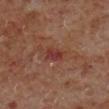Q: Is there a histopathology result?
A: imaged on a skin check; not biopsied
Q: What are the patient's age and sex?
A: male, about 60 years old
Q: Automated lesion metrics?
A: a classifier nevus-likeness of about 0/100 and a lesion-detection confidence of about 100/100
Q: What lighting was used for the tile?
A: cross-polarized illumination
Q: How was this image acquired?
A: total-body-photography crop, ~15 mm field of view
Q: What is the lesion's diameter?
A: ≈2.5 mm
Q: Lesion location?
A: the right lower leg